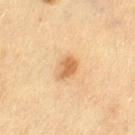workup: imaged on a skin check; not biopsied
patient: female, aged 53–57
imaging modality: 15 mm crop, total-body photography
body site: the leg
size: about 3 mm
automated lesion analysis: an eccentricity of roughly 0.75 and a shape-asymmetry score of about 0.3 (0 = symmetric); an average lesion color of about L≈57 a*≈19 b*≈37 (CIELAB); an automated nevus-likeness rating near 90 out of 100 and a detector confidence of about 100 out of 100 that the crop contains a lesion
illumination: cross-polarized